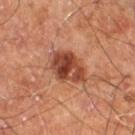Clinical impression: The lesion was tiled from a total-body skin photograph and was not biopsied. Clinical summary: A 15 mm close-up extracted from a 3D total-body photography capture. On the right thigh. Longest diameter approximately 5 mm. A male subject, in their 60s. This is a cross-polarized tile.